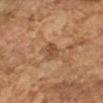biopsy_status: not biopsied; imaged during a skin examination
patient:
  sex: male
  age_approx: 60
site: left forearm
lesion_size:
  long_diameter_mm_approx: 3.0
lighting: cross-polarized
image:
  source: total-body photography crop
  field_of_view_mm: 15